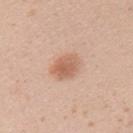Recorded during total-body skin imaging; not selected for excision or biopsy. A region of skin cropped from a whole-body photographic capture, roughly 15 mm wide. The lesion is on the left upper arm. A female subject aged 18 to 22.